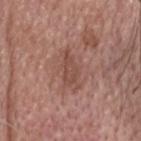The lesion was photographed on a routine skin check and not biopsied; there is no pathology result.
The tile uses white-light illumination.
Located on the head or neck.
The recorded lesion diameter is about 4 mm.
The patient is a male aged 73 to 77.
A lesion tile, about 15 mm wide, cut from a 3D total-body photograph.